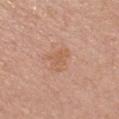No biopsy was performed on this lesion — it was imaged during a full skin examination and was not determined to be concerning.
A close-up tile cropped from a whole-body skin photograph, about 15 mm across.
Measured at roughly 3 mm in maximum diameter.
The tile uses white-light illumination.
On the chest.
A female subject, aged approximately 55.